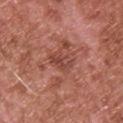From the upper back.
Cropped from a whole-body photographic skin survey; the tile spans about 15 mm.
The subject is a male aged approximately 65.